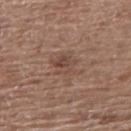Assessment: The lesion was tiled from a total-body skin photograph and was not biopsied. Background: Located on the back. A female patient, in their mid-60s. This image is a 15 mm lesion crop taken from a total-body photograph.